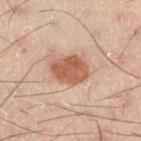follow-up — no biopsy performed (imaged during a skin exam)
image source — 15 mm crop, total-body photography
diameter — about 4 mm
subject — male, aged approximately 50
site — the right thigh